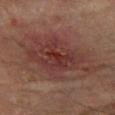Q: Is there a histopathology result?
A: no biopsy performed (imaged during a skin exam)
Q: What are the patient's age and sex?
A: male, about 45 years old
Q: How was the tile lit?
A: cross-polarized
Q: Lesion location?
A: the right forearm
Q: Lesion size?
A: ~9 mm (longest diameter)
Q: How was this image acquired?
A: 15 mm crop, total-body photography